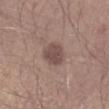Impression: Captured during whole-body skin photography for melanoma surveillance; the lesion was not biopsied. Acquisition and patient details: From the right forearm. This is a white-light tile. About 3 mm across. A male patient, aged approximately 45. This image is a 15 mm lesion crop taken from a total-body photograph.